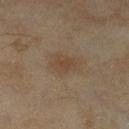- workup — no biopsy performed (imaged during a skin exam)
- lesion size — ~2.5 mm (longest diameter)
- acquisition — ~15 mm crop, total-body skin-cancer survey
- image-analysis metrics — a lesion area of about 4 mm², an outline eccentricity of about 0.65 (0 = round, 1 = elongated), and a shape-asymmetry score of about 0.3 (0 = symmetric)
- anatomic site — the left lower leg
- illumination — cross-polarized
- subject — female, in their 60s